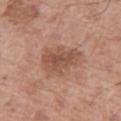Assessment:
Imaged during a routine full-body skin examination; the lesion was not biopsied and no histopathology is available.
Context:
On the left thigh. This image is a 15 mm lesion crop taken from a total-body photograph. Automated image analysis of the tile measured a lesion area of about 11 mm² and a symmetry-axis asymmetry near 0.25. The analysis additionally found border irregularity of about 3.5 on a 0–10 scale. The analysis additionally found a detector confidence of about 100 out of 100 that the crop contains a lesion. About 4.5 mm across. The subject is a male approximately 70 years of age. Captured under white-light illumination.